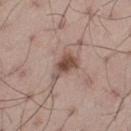biopsy status — total-body-photography surveillance lesion; no biopsy | acquisition — total-body-photography crop, ~15 mm field of view | patient — male, about 50 years old | lighting — white-light illumination | lesion size — about 4 mm | anatomic site — the leg.